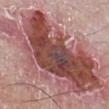The lesion was tiled from a total-body skin photograph and was not biopsied.
From the right lower leg.
About 13.5 mm across.
Automated tile analysis of the lesion measured border irregularity of about 7.5 on a 0–10 scale, a within-lesion color-variation index near 9.5/10, and peripheral color asymmetry of about 3.
The subject is a male aged approximately 50.
Captured under white-light illumination.
A region of skin cropped from a whole-body photographic capture, roughly 15 mm wide.